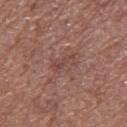  biopsy_status: not biopsied; imaged during a skin examination
  image:
    source: total-body photography crop
    field_of_view_mm: 15
  lighting: white-light
  lesion_size:
    long_diameter_mm_approx: 3.0
  patient:
    sex: male
    age_approx: 60
  site: upper back
  automated_metrics:
    cielab_L: 45
    cielab_a: 21
    cielab_b: 24
    vs_skin_darker_L: 6.0
    vs_skin_contrast_norm: 5.0
    border_irregularity_0_10: 5.0
    peripheral_color_asymmetry: 0.0
    nevus_likeness_0_100: 0
    lesion_detection_confidence_0_100: 95